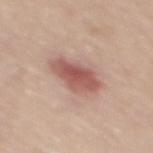This lesion was catalogued during total-body skin photography and was not selected for biopsy. The subject is a female aged 48–52. The lesion's longest dimension is about 5 mm. A region of skin cropped from a whole-body photographic capture, roughly 15 mm wide. The lesion is located on the back. This is a white-light tile.